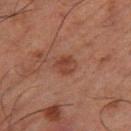{"biopsy_status": "not biopsied; imaged during a skin examination", "site": "right thigh", "patient": {"sex": "male", "age_approx": 65}, "image": {"source": "total-body photography crop", "field_of_view_mm": 15}, "automated_metrics": {"border_irregularity_0_10": 1.5, "color_variation_0_10": 2.5}}